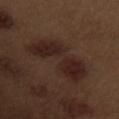Assessment: The lesion was tiled from a total-body skin photograph and was not biopsied. Background: A male patient approximately 70 years of age. The lesion's longest dimension is about 7.5 mm. A lesion tile, about 15 mm wide, cut from a 3D total-body photograph. The lesion is located on the left upper arm.